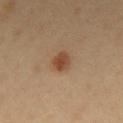From the back.
The subject is a male about 50 years old.
A roughly 15 mm field-of-view crop from a total-body skin photograph.